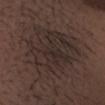workup: imaged on a skin check; not biopsied | acquisition: ~15 mm crop, total-body skin-cancer survey | diameter: ~9 mm (longest diameter) | patient: male, aged 28 to 32 | body site: the head or neck.